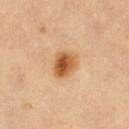Q: Is there a histopathology result?
A: no biopsy performed (imaged during a skin exam)
Q: Who is the patient?
A: female, roughly 70 years of age
Q: Automated lesion metrics?
A: a lesion color around L≈59 a*≈24 b*≈42 in CIELAB, about 15 CIELAB-L* units darker than the surrounding skin, and a normalized border contrast of about 10; a border-irregularity index near 1.5/10, a color-variation rating of about 7/10, and peripheral color asymmetry of about 2
Q: What kind of image is this?
A: 15 mm crop, total-body photography
Q: Lesion location?
A: the right thigh
Q: Illumination type?
A: cross-polarized illumination
Q: Lesion size?
A: ≈3.5 mm